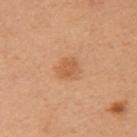follow-up — catalogued during a skin exam; not biopsied.